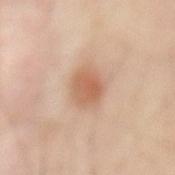From the mid back.
The recorded lesion diameter is about 3.5 mm.
The subject is aged around 55.
A 15 mm crop from a total-body photograph taken for skin-cancer surveillance.